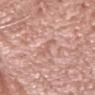The lesion was tiled from a total-body skin photograph and was not biopsied.
Cropped from a total-body skin-imaging series; the visible field is about 15 mm.
This is a white-light tile.
The lesion's longest dimension is about 2.5 mm.
The lesion is on the head or neck.
A male patient, aged 58 to 62.